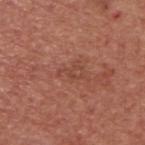Case summary:
- workup · catalogued during a skin exam; not biopsied
- tile lighting · white-light
- subject · male, roughly 65 years of age
- lesion diameter · about 3 mm
- site · the upper back
- acquisition · ~15 mm crop, total-body skin-cancer survey
- automated lesion analysis · a lesion color around L≈45 a*≈25 b*≈30 in CIELAB, about 6 CIELAB-L* units darker than the surrounding skin, and a lesion-to-skin contrast of about 4.5 (normalized; higher = more distinct); a color-variation rating of about 1.5/10 and peripheral color asymmetry of about 0.5